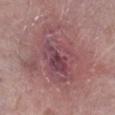Captured during whole-body skin photography for melanoma surveillance; the lesion was not biopsied. The patient is a female approximately 60 years of age. From the right lower leg. Imaged with white-light lighting. A lesion tile, about 15 mm wide, cut from a 3D total-body photograph.